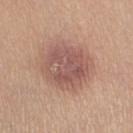Findings:
- lesion diameter · about 5.5 mm
- subject · female, aged 58 to 62
- illumination · white-light illumination
- image source · total-body-photography crop, ~15 mm field of view
- image-analysis metrics · roughly 10 lightness units darker than nearby skin and a normalized border contrast of about 7.5; an automated nevus-likeness rating near 55 out of 100 and a lesion-detection confidence of about 100/100
- body site · the left thigh